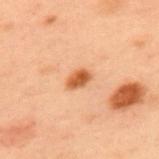<record>
<biopsy_status>not biopsied; imaged during a skin examination</biopsy_status>
<lighting>cross-polarized</lighting>
<image>
  <source>total-body photography crop</source>
  <field_of_view_mm>15</field_of_view_mm>
</image>
<patient>
  <sex>male</sex>
  <age_approx>55</age_approx>
</patient>
<site>upper back</site>
<lesion_size>
  <long_diameter_mm_approx>2.5</long_diameter_mm_approx>
</lesion_size>
</record>